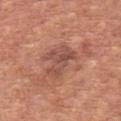Part of a total-body skin-imaging series; this lesion was reviewed on a skin check and was not flagged for biopsy. The total-body-photography lesion software estimated a shape eccentricity near 0.8. A male subject in their mid- to late 60s. The recorded lesion diameter is about 5 mm. A lesion tile, about 15 mm wide, cut from a 3D total-body photograph. On the chest. This is a white-light tile.